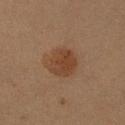Q: Is there a histopathology result?
A: imaged on a skin check; not biopsied
Q: Where on the body is the lesion?
A: the right upper arm
Q: What kind of image is this?
A: ~15 mm crop, total-body skin-cancer survey
Q: What is the lesion's diameter?
A: ~3.5 mm (longest diameter)
Q: Who is the patient?
A: female, approximately 40 years of age